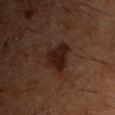{"biopsy_status": "not biopsied; imaged during a skin examination", "site": "chest", "lighting": "cross-polarized", "patient": {"sex": "male", "age_approx": 50}, "automated_metrics": {"eccentricity": 0.45, "shape_asymmetry": 0.3, "cielab_L": 14, "cielab_a": 14, "cielab_b": 16, "vs_skin_darker_L": 7.0, "vs_skin_contrast_norm": 10.5, "nevus_likeness_0_100": 90}, "image": {"source": "total-body photography crop", "field_of_view_mm": 15}}